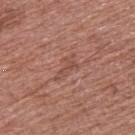notes: catalogued during a skin exam; not biopsied | image-analysis metrics: a border-irregularity rating of about 6.5/10 and peripheral color asymmetry of about 0; a classifier nevus-likeness of about 0/100 and lesion-presence confidence of about 75/100 | tile lighting: white-light illumination | subject: male, aged approximately 55 | site: the upper back | diameter: ~3.5 mm (longest diameter) | image source: ~15 mm crop, total-body skin-cancer survey.